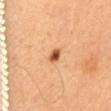The lesion-visualizer software estimated a lesion area of about 3 mm², an eccentricity of roughly 0.8, and a shape-asymmetry score of about 0.2 (0 = symmetric). And it measured an average lesion color of about L≈57 a*≈28 b*≈42 (CIELAB) and roughly 17 lightness units darker than nearby skin. The analysis additionally found an automated nevus-likeness rating near 100 out of 100 and lesion-presence confidence of about 100/100. Captured under cross-polarized illumination. Cropped from a whole-body photographic skin survey; the tile spans about 15 mm. A female subject, about 30 years old. From the mid back.